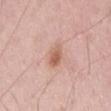notes: no biopsy performed (imaged during a skin exam).